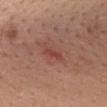Imaged during a routine full-body skin examination; the lesion was not biopsied and no histopathology is available. About 3 mm across. The tile uses white-light illumination. The patient is a female aged approximately 50. A region of skin cropped from a whole-body photographic capture, roughly 15 mm wide. Located on the chest. The total-body-photography lesion software estimated border irregularity of about 3 on a 0–10 scale, internal color variation of about 1 on a 0–10 scale, and peripheral color asymmetry of about 0.